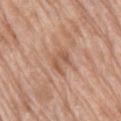workup: total-body-photography surveillance lesion; no biopsy | subject: female, about 75 years old | location: the right thigh | acquisition: ~15 mm crop, total-body skin-cancer survey.